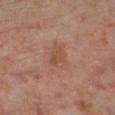Findings:
– workup: total-body-photography surveillance lesion; no biopsy
– body site: the leg
– size: ≈3 mm
– image: 15 mm crop, total-body photography
– automated lesion analysis: a footprint of about 5 mm² and an eccentricity of roughly 0.7; radial color variation of about 1; a nevus-likeness score of about 0/100
– patient: male, aged 58 to 62